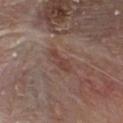Impression: This lesion was catalogued during total-body skin photography and was not selected for biopsy. Background: The lesion is on the front of the torso. A lesion tile, about 15 mm wide, cut from a 3D total-body photograph. Captured under white-light illumination. A male subject, roughly 80 years of age. An algorithmic analysis of the crop reported a footprint of about 3.5 mm², an outline eccentricity of about 0.95 (0 = round, 1 = elongated), and a symmetry-axis asymmetry near 0.4. And it measured a classifier nevus-likeness of about 0/100. Measured at roughly 3.5 mm in maximum diameter.